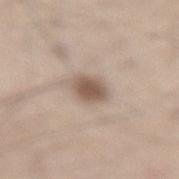The tile uses white-light illumination. The lesion's longest dimension is about 3 mm. Located on the lower back. The total-body-photography lesion software estimated a footprint of about 6 mm², a shape eccentricity near 0.65, and a shape-asymmetry score of about 0.2 (0 = symmetric). And it measured an average lesion color of about L≈55 a*≈15 b*≈25 (CIELAB), about 13 CIELAB-L* units darker than the surrounding skin, and a normalized lesion–skin contrast near 9. The analysis additionally found a border-irregularity rating of about 2/10, a color-variation rating of about 2.5/10, and peripheral color asymmetry of about 0.5. Cropped from a whole-body photographic skin survey; the tile spans about 15 mm. A male patient, roughly 55 years of age.